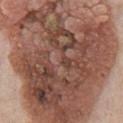This lesion was catalogued during total-body skin photography and was not selected for biopsy.
A male subject aged approximately 75.
A close-up tile cropped from a whole-body skin photograph, about 15 mm across.
The lesion is located on the chest.